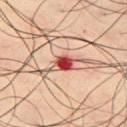Q: Was this lesion biopsied?
A: total-body-photography surveillance lesion; no biopsy
Q: What is the imaging modality?
A: 15 mm crop, total-body photography
Q: What is the lesion's diameter?
A: ~3 mm (longest diameter)
Q: Who is the patient?
A: male, in their 50s
Q: What lighting was used for the tile?
A: cross-polarized
Q: Where on the body is the lesion?
A: the chest